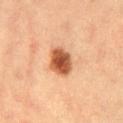tile lighting = cross-polarized illumination | patient = female, aged around 50 | image source = 15 mm crop, total-body photography.